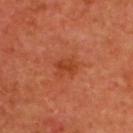Impression: Captured during whole-body skin photography for melanoma surveillance; the lesion was not biopsied. Image and clinical context: A female subject roughly 45 years of age. A 15 mm crop from a total-body photograph taken for skin-cancer surveillance. The lesion is on the upper back. An algorithmic analysis of the crop reported an outline eccentricity of about 0.75 (0 = round, 1 = elongated) and a symmetry-axis asymmetry near 0.25. And it measured a border-irregularity index near 2.5/10, a within-lesion color-variation index near 2/10, and a peripheral color-asymmetry measure near 0.5. The lesion's longest dimension is about 2.5 mm. Imaged with cross-polarized lighting.